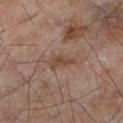The lesion was photographed on a routine skin check and not biopsied; there is no pathology result.
A male subject about 65 years old.
A 15 mm crop from a total-body photograph taken for skin-cancer surveillance.
On the left lower leg.
This is a cross-polarized tile.
The total-body-photography lesion software estimated an area of roughly 3.5 mm², a shape eccentricity near 0.9, and a shape-asymmetry score of about 0.45 (0 = symmetric). It also reported a border-irregularity index near 5/10, a color-variation rating of about 1.5/10, and a peripheral color-asymmetry measure near 0. The software also gave an automated nevus-likeness rating near 0 out of 100 and lesion-presence confidence of about 100/100.